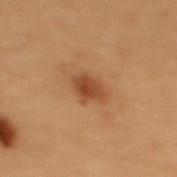| key | value |
|---|---|
| workup | no biopsy performed (imaged during a skin exam) |
| lighting | cross-polarized illumination |
| diameter | ≈3 mm |
| anatomic site | the upper back |
| acquisition | total-body-photography crop, ~15 mm field of view |
| subject | female, in their 50s |